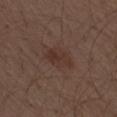Impression:
No biopsy was performed on this lesion — it was imaged during a full skin examination and was not determined to be concerning.
Context:
A lesion tile, about 15 mm wide, cut from a 3D total-body photograph. A male subject, approximately 70 years of age. From the right thigh.